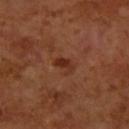* biopsy status — no biopsy performed (imaged during a skin exam)